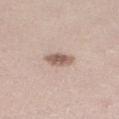The lesion was tiled from a total-body skin photograph and was not biopsied. Longest diameter approximately 3.5 mm. Imaged with white-light lighting. Cropped from a whole-body photographic skin survey; the tile spans about 15 mm. The lesion is located on the right lower leg. The subject is a female approximately 40 years of age.